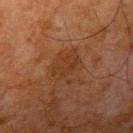{"biopsy_status": "not biopsied; imaged during a skin examination", "automated_metrics": {"area_mm2_approx": 4.5, "shape_asymmetry": 0.45}, "patient": {"sex": "male", "age_approx": 80}, "site": "right upper arm", "lighting": "cross-polarized", "image": {"source": "total-body photography crop", "field_of_view_mm": 15}}